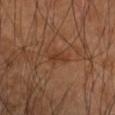Findings:
* anatomic site — the right forearm
* acquisition — ~15 mm crop, total-body skin-cancer survey
* TBP lesion metrics — a lesion area of about 2.5 mm² and an outline eccentricity of about 0.9 (0 = round, 1 = elongated); a lesion–skin lightness drop of about 8 and a lesion-to-skin contrast of about 7 (normalized; higher = more distinct)